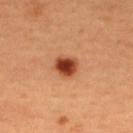<tbp_lesion>
<lighting>cross-polarized</lighting>
<site>upper back</site>
<lesion_size>
  <long_diameter_mm_approx>3.0</long_diameter_mm_approx>
</lesion_size>
<patient>
  <sex>female</sex>
  <age_approx>50</age_approx>
</patient>
<image>
  <source>total-body photography crop</source>
  <field_of_view_mm>15</field_of_view_mm>
</image>
<diagnosis>
  <histopathology>atypical melanocytic neoplasm</histopathology>
  <malignancy>indeterminate</malignancy>
  <taxonomic_path>Indeterminate, Indeterminate melanocytic proliferations, Atypical melanocytic neoplasm</taxonomic_path>
</diagnosis>
</tbp_lesion>